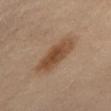biopsy_status: not biopsied; imaged during a skin examination
lighting: cross-polarized
site: mid back
patient:
  sex: female
  age_approx: 60
lesion_size:
  long_diameter_mm_approx: 6.0
image:
  source: total-body photography crop
  field_of_view_mm: 15